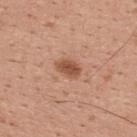* illumination · white-light illumination
* TBP lesion metrics · a lesion area of about 5 mm², a shape eccentricity near 0.75, and two-axis asymmetry of about 0.2; a mean CIELAB color near L≈53 a*≈24 b*≈32, a lesion–skin lightness drop of about 11, and a normalized lesion–skin contrast near 8
* imaging modality · total-body-photography crop, ~15 mm field of view
* subject · male, roughly 30 years of age
* body site · the upper back